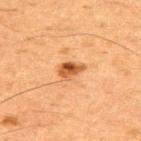* notes — imaged on a skin check; not biopsied
* body site — the upper back
* tile lighting — cross-polarized illumination
* patient — male, roughly 65 years of age
* image source — 15 mm crop, total-body photography
* lesion diameter — about 3 mm